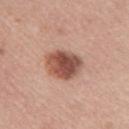follow-up: imaged on a skin check; not biopsied | lesion diameter: ~4.5 mm (longest diameter) | image: ~15 mm crop, total-body skin-cancer survey | image-analysis metrics: a lesion area of about 12 mm² and an eccentricity of roughly 0.45; a mean CIELAB color near L≈51 a*≈23 b*≈28 and roughly 16 lightness units darker than nearby skin | body site: the right upper arm | lighting: white-light illumination | subject: female, aged approximately 60.